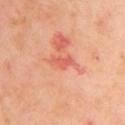<tbp_lesion>
  <biopsy_status>not biopsied; imaged during a skin examination</biopsy_status>
  <patient>
    <sex>female</sex>
    <age_approx>55</age_approx>
  </patient>
  <automated_metrics>
    <eccentricity>0.9</eccentricity>
    <shape_asymmetry>0.35</shape_asymmetry>
    <lesion_detection_confidence_0_100>100</lesion_detection_confidence_0_100>
  </automated_metrics>
  <image>
    <source>total-body photography crop</source>
    <field_of_view_mm>15</field_of_view_mm>
  </image>
  <lesion_size>
    <long_diameter_mm_approx>3.5</long_diameter_mm_approx>
  </lesion_size>
  <site>arm</site>
</tbp_lesion>